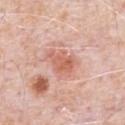lighting: white-light
image:
  source: total-body photography crop
  field_of_view_mm: 15
site: chest
automated_metrics:
  area_mm2_approx: 6.0
  eccentricity: 0.7
  shape_asymmetry: 0.3
  cielab_L: 61
  cielab_a: 26
  cielab_b: 31
  vs_skin_darker_L: 10.0
  vs_skin_contrast_norm: 7.0
  nevus_likeness_0_100: 0
  lesion_detection_confidence_0_100: 100
patient:
  sex: male
  age_approx: 80